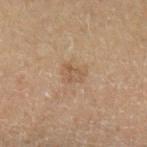| key | value |
|---|---|
| notes | total-body-photography surveillance lesion; no biopsy |
| lesion size | ≈2.5 mm |
| image | ~15 mm tile from a whole-body skin photo |
| illumination | cross-polarized |
| body site | the leg |
| subject | male, in their 70s |